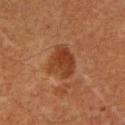The lesion was photographed on a routine skin check and not biopsied; there is no pathology result. Measured at roughly 4.5 mm in maximum diameter. Cropped from a total-body skin-imaging series; the visible field is about 15 mm. A male subject roughly 60 years of age. An algorithmic analysis of the crop reported a nevus-likeness score of about 65/100 and a detector confidence of about 100 out of 100 that the crop contains a lesion. On the right upper arm.